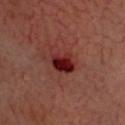Context:
The subject is about 65 years old. Located on the head or neck. An algorithmic analysis of the crop reported an area of roughly 8 mm², a shape eccentricity near 0.3, and two-axis asymmetry of about 0.2. The software also gave an average lesion color of about L≈27 a*≈29 b*≈24 (CIELAB), a lesion–skin lightness drop of about 12, and a normalized lesion–skin contrast near 11. It also reported a nevus-likeness score of about 0/100. A region of skin cropped from a whole-body photographic capture, roughly 15 mm wide. The lesion's longest dimension is about 3.5 mm.